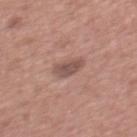Clinical impression: No biopsy was performed on this lesion — it was imaged during a full skin examination and was not determined to be concerning. Clinical summary: Automated tile analysis of the lesion measured an area of roughly 5 mm², an eccentricity of roughly 0.75, and a symmetry-axis asymmetry near 0.25. And it measured an average lesion color of about L≈51 a*≈19 b*≈23 (CIELAB) and about 10 CIELAB-L* units darker than the surrounding skin. It also reported a within-lesion color-variation index near 2.5/10 and radial color variation of about 0.5. The lesion's longest dimension is about 3 mm. Cropped from a whole-body photographic skin survey; the tile spans about 15 mm. Located on the chest. The subject is a male aged 43–47.